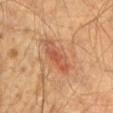{"biopsy_status": "not biopsied; imaged during a skin examination", "lesion_size": {"long_diameter_mm_approx": 5.5}, "automated_metrics": {"area_mm2_approx": 8.0, "shape_asymmetry": 0.25, "cielab_L": 48, "cielab_a": 23, "cielab_b": 32, "vs_skin_darker_L": 9.0, "color_variation_0_10": 4.0, "lesion_detection_confidence_0_100": 100}, "site": "chest", "image": {"source": "total-body photography crop", "field_of_view_mm": 15}, "lighting": "cross-polarized", "patient": {"sex": "male", "age_approx": 65}}